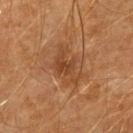notes: catalogued during a skin exam; not biopsied | patient: male, aged 58–62 | anatomic site: the arm | diameter: about 5 mm | TBP lesion metrics: an outline eccentricity of about 0.8 (0 = round, 1 = elongated); an average lesion color of about L≈42 a*≈22 b*≈34 (CIELAB), about 8 CIELAB-L* units darker than the surrounding skin, and a normalized border contrast of about 6.5; an automated nevus-likeness rating near 10 out of 100 and a detector confidence of about 100 out of 100 that the crop contains a lesion | acquisition: 15 mm crop, total-body photography | tile lighting: cross-polarized illumination.